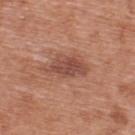Notes:
• workup — imaged on a skin check; not biopsied
• image source — 15 mm crop, total-body photography
• location — the upper back
• automated lesion analysis — an eccentricity of roughly 0.8; an average lesion color of about L≈49 a*≈24 b*≈28 (CIELAB) and a normalized lesion–skin contrast near 7.5; a nevus-likeness score of about 55/100
• patient — male, roughly 70 years of age
• tile lighting — white-light
• size — ~5 mm (longest diameter)